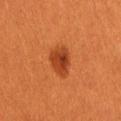The lesion is located on the lower back. Captured under cross-polarized illumination. The patient is a female aged 28–32. A 15 mm close-up tile from a total-body photography series done for melanoma screening.